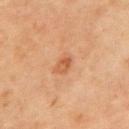biopsy status = catalogued during a skin exam; not biopsied
acquisition = ~15 mm crop, total-body skin-cancer survey
patient = female, aged 68–72
lesion size = ~3 mm (longest diameter)
automated metrics = a classifier nevus-likeness of about 35/100 and lesion-presence confidence of about 100/100
anatomic site = the left upper arm